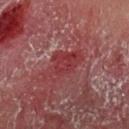{"biopsy_status": "not biopsied; imaged during a skin examination", "site": "left upper arm", "image": {"source": "total-body photography crop", "field_of_view_mm": 15}, "patient": {"sex": "male", "age_approx": 55}, "automated_metrics": {"area_mm2_approx": 10.0, "shape_asymmetry": 0.35, "cielab_L": 33, "cielab_a": 29, "cielab_b": 20, "vs_skin_darker_L": 6.0, "vs_skin_contrast_norm": 6.5, "lesion_detection_confidence_0_100": 25}, "lesion_size": {"long_diameter_mm_approx": 4.5}, "lighting": "cross-polarized"}